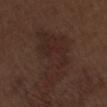– notes — total-body-photography surveillance lesion; no biopsy
– subject — male, aged around 70
– image — ~15 mm crop, total-body skin-cancer survey
– site — the lower back
– tile lighting — white-light
– size — ~9 mm (longest diameter)
– TBP lesion metrics — a footprint of about 21 mm² and two-axis asymmetry of about 0.5; border irregularity of about 8 on a 0–10 scale, a color-variation rating of about 4/10, and a peripheral color-asymmetry measure near 1.5